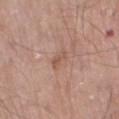Q: Was a biopsy performed?
A: catalogued during a skin exam; not biopsied
Q: Where on the body is the lesion?
A: the leg
Q: Automated lesion metrics?
A: an automated nevus-likeness rating near 0 out of 100 and a lesion-detection confidence of about 100/100
Q: Who is the patient?
A: male, aged approximately 80
Q: How was this image acquired?
A: ~15 mm crop, total-body skin-cancer survey
Q: What is the lesion's diameter?
A: ~2.5 mm (longest diameter)
Q: What lighting was used for the tile?
A: white-light